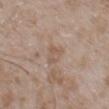Notes:
– follow-up · total-body-photography surveillance lesion; no biopsy
– subject · male, aged approximately 50
– site · the back
– diameter · about 2.5 mm
– acquisition · 15 mm crop, total-body photography
– TBP lesion metrics · a mean CIELAB color near L≈56 a*≈15 b*≈27, about 6 CIELAB-L* units darker than the surrounding skin, and a lesion-to-skin contrast of about 5 (normalized; higher = more distinct)